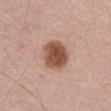image: total-body-photography crop, ~15 mm field of view; diameter: ≈4 mm; location: the abdomen; subject: male, aged around 60; illumination: white-light illumination.